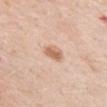Captured during whole-body skin photography for melanoma surveillance; the lesion was not biopsied. About 2.5 mm across. The patient is a female roughly 60 years of age. The lesion is on the chest. Automated image analysis of the tile measured a footprint of about 4.5 mm² and a shape-asymmetry score of about 0.2 (0 = symmetric). And it measured a lesion–skin lightness drop of about 11. The software also gave a border-irregularity index near 2/10. The software also gave an automated nevus-likeness rating near 90 out of 100 and lesion-presence confidence of about 100/100. Cropped from a total-body skin-imaging series; the visible field is about 15 mm. This is a white-light tile.